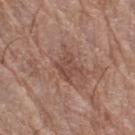notes = imaged on a skin check; not biopsied | body site = the right thigh | acquisition = ~15 mm tile from a whole-body skin photo | lesion diameter = about 3 mm | automated metrics = border irregularity of about 2.5 on a 0–10 scale and peripheral color asymmetry of about 1; a nevus-likeness score of about 0/100 and a lesion-detection confidence of about 95/100 | patient = female, aged around 80 | tile lighting = white-light.